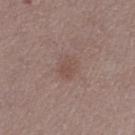workup — total-body-photography surveillance lesion; no biopsy
body site — the left thigh
lighting — white-light illumination
image — total-body-photography crop, ~15 mm field of view
patient — female, aged 48–52
diameter — about 3 mm